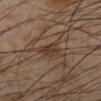Q: Was this lesion biopsied?
A: imaged on a skin check; not biopsied
Q: Automated lesion metrics?
A: roughly 7 lightness units darker than nearby skin and a normalized border contrast of about 7
Q: What lighting was used for the tile?
A: cross-polarized illumination
Q: Who is the patient?
A: male, in their mid-50s
Q: What is the anatomic site?
A: the left lower leg
Q: What kind of image is this?
A: ~15 mm crop, total-body skin-cancer survey
Q: How large is the lesion?
A: ~2.5 mm (longest diameter)